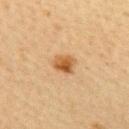| key | value |
|---|---|
| illumination | cross-polarized illumination |
| lesion size | ~2.5 mm (longest diameter) |
| acquisition | total-body-photography crop, ~15 mm field of view |
| body site | the upper back |
| subject | female, in their mid- to late 40s |
| automated metrics | an area of roughly 5 mm² and a shape eccentricity near 0.5; an average lesion color of about L≈50 a*≈20 b*≈38 (CIELAB) and roughly 11 lightness units darker than nearby skin; a border-irregularity index near 2/10, a color-variation rating of about 5/10, and peripheral color asymmetry of about 2; an automated nevus-likeness rating near 95 out of 100 and a detector confidence of about 100 out of 100 that the crop contains a lesion |